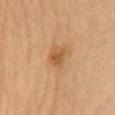Findings:
- biopsy status — imaged on a skin check; not biopsied
- acquisition — ~15 mm crop, total-body skin-cancer survey
- lighting — cross-polarized illumination
- subject — female, in their 60s
- site — the chest